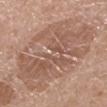{"biopsy_status": "not biopsied; imaged during a skin examination", "lesion_size": {"long_diameter_mm_approx": 11.5}, "patient": {"sex": "female", "age_approx": 55}, "automated_metrics": {"border_irregularity_0_10": 2.5, "color_variation_0_10": 6.0}, "site": "right forearm", "image": {"source": "total-body photography crop", "field_of_view_mm": 15}, "lighting": "white-light"}